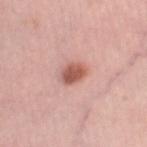notes: imaged on a skin check; not biopsied | body site: the leg | image source: 15 mm crop, total-body photography | patient: female, aged around 45.